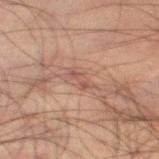The lesion was photographed on a routine skin check and not biopsied; there is no pathology result.
The lesion's longest dimension is about 3 mm.
On the leg.
A male subject in their 50s.
Cropped from a total-body skin-imaging series; the visible field is about 15 mm.